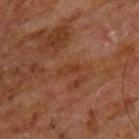biopsy status = total-body-photography surveillance lesion; no biopsy
illumination = cross-polarized
TBP lesion metrics = a lesion area of about 2.5 mm² and an eccentricity of roughly 0.9; radial color variation of about 0
body site = the chest
image = 15 mm crop, total-body photography
patient = male, in their 60s
lesion size = ≈3 mm